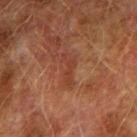The lesion was photographed on a routine skin check and not biopsied; there is no pathology result.
A lesion tile, about 15 mm wide, cut from a 3D total-body photograph.
Measured at roughly 3.5 mm in maximum diameter.
The subject is a male approximately 75 years of age.
On the right upper arm.
This is a cross-polarized tile.